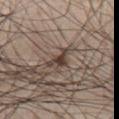Acquisition and patient details:
This is a white-light tile. Cropped from a total-body skin-imaging series; the visible field is about 15 mm. The total-body-photography lesion software estimated an area of roughly 4.5 mm² and a shape-asymmetry score of about 0.65 (0 = symmetric). Located on the chest. A male subject aged around 35. The recorded lesion diameter is about 3.5 mm.